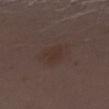follow-up=no biopsy performed (imaged during a skin exam)
image-analysis metrics=a mean CIELAB color near L≈31 a*≈14 b*≈20 and a normalized border contrast of about 5; border irregularity of about 3 on a 0–10 scale and a peripheral color-asymmetry measure near 0.5
lesion size=≈2.5 mm
body site=the arm
acquisition=15 mm crop, total-body photography
tile lighting=white-light
patient=female, aged approximately 30